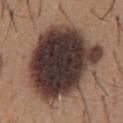Findings:
- biopsy status — imaged on a skin check; not biopsied
- image — ~15 mm tile from a whole-body skin photo
- illumination — white-light
- diameter — ~10 mm (longest diameter)
- patient — male, aged 63–67
- anatomic site — the chest